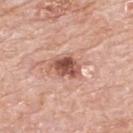Recorded during total-body skin imaging; not selected for excision or biopsy.
This is a white-light tile.
The recorded lesion diameter is about 3.5 mm.
A roughly 15 mm field-of-view crop from a total-body skin photograph.
A male patient, approximately 80 years of age.
From the back.